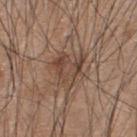This lesion was catalogued during total-body skin photography and was not selected for biopsy. This is a white-light tile. Cropped from a total-body skin-imaging series; the visible field is about 15 mm. Located on the upper back. A male patient, roughly 45 years of age.